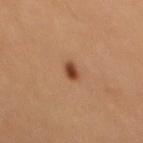Notes:
– follow-up: no biopsy performed (imaged during a skin exam)
– subject: male, aged around 65
– illumination: cross-polarized illumination
– size: ≈2 mm
– image source: 15 mm crop, total-body photography
– location: the mid back